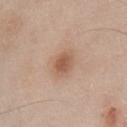Q: Is there a histopathology result?
A: no biopsy performed (imaged during a skin exam)
Q: Lesion size?
A: ~2.5 mm (longest diameter)
Q: What lighting was used for the tile?
A: white-light illumination
Q: What kind of image is this?
A: total-body-photography crop, ~15 mm field of view
Q: Who is the patient?
A: male, roughly 55 years of age
Q: Where on the body is the lesion?
A: the chest